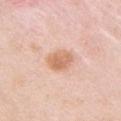Captured during whole-body skin photography for melanoma surveillance; the lesion was not biopsied.
The lesion's longest dimension is about 3.5 mm.
The subject is a female aged approximately 65.
On the left upper arm.
A region of skin cropped from a whole-body photographic capture, roughly 15 mm wide.
Imaged with white-light lighting.
Automated image analysis of the tile measured a lesion area of about 7 mm², an eccentricity of roughly 0.7, and two-axis asymmetry of about 0.2. It also reported roughly 11 lightness units darker than nearby skin and a normalized border contrast of about 7.5. And it measured a border-irregularity index near 2/10, internal color variation of about 3.5 on a 0–10 scale, and radial color variation of about 1.